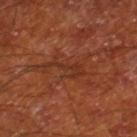Impression:
Captured during whole-body skin photography for melanoma surveillance; the lesion was not biopsied.
Clinical summary:
The subject is a male approximately 65 years of age. On the right lower leg. This image is a 15 mm lesion crop taken from a total-body photograph.